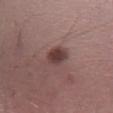{"biopsy_status": "not biopsied; imaged during a skin examination", "patient": {"sex": "male", "age_approx": 25}, "image": {"source": "total-body photography crop", "field_of_view_mm": 15}, "lighting": "white-light", "lesion_size": {"long_diameter_mm_approx": 3.0}, "site": "right lower leg"}